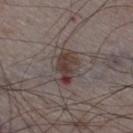notes: total-body-photography surveillance lesion; no biopsy
location: the right thigh
patient: male, in their mid- to late 70s
acquisition: 15 mm crop, total-body photography